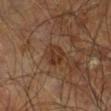Assessment: Captured during whole-body skin photography for melanoma surveillance; the lesion was not biopsied. Image and clinical context: The lesion is located on the leg. A male subject aged approximately 60. Cropped from a total-body skin-imaging series; the visible field is about 15 mm.